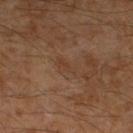Assessment:
Imaged during a routine full-body skin examination; the lesion was not biopsied and no histopathology is available.
Background:
A male patient aged approximately 60. The total-body-photography lesion software estimated an area of roughly 2.5 mm², a shape eccentricity near 0.9, and a shape-asymmetry score of about 0.35 (0 = symmetric). The analysis additionally found a mean CIELAB color near L≈34 a*≈16 b*≈27 and a normalized border contrast of about 4.5. The recorded lesion diameter is about 2.5 mm. Cropped from a total-body skin-imaging series; the visible field is about 15 mm. The tile uses cross-polarized illumination. The lesion is on the left lower leg.